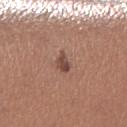Q: What are the patient's age and sex?
A: female, roughly 35 years of age
Q: What kind of image is this?
A: ~15 mm tile from a whole-body skin photo
Q: What did automated image analysis measure?
A: a lesion area of about 3.5 mm², a shape eccentricity near 0.75, and a symmetry-axis asymmetry near 0.3; a lesion color around L≈46 a*≈21 b*≈24 in CIELAB and about 11 CIELAB-L* units darker than the surrounding skin; an automated nevus-likeness rating near 50 out of 100 and a detector confidence of about 100 out of 100 that the crop contains a lesion
Q: What is the lesion's diameter?
A: ~2.5 mm (longest diameter)
Q: Lesion location?
A: the left lower leg
Q: What lighting was used for the tile?
A: white-light